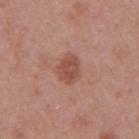workup: imaged on a skin check; not biopsied | patient: female, aged 38–42 | image source: ~15 mm crop, total-body skin-cancer survey | body site: the arm.